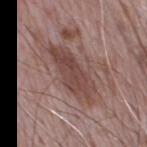No biopsy was performed on this lesion — it was imaged during a full skin examination and was not determined to be concerning. On the chest. About 8 mm across. An algorithmic analysis of the crop reported a mean CIELAB color near L≈46 a*≈19 b*≈21, a lesion–skin lightness drop of about 10, and a normalized border contrast of about 7.5. It also reported an automated nevus-likeness rating near 10 out of 100 and a lesion-detection confidence of about 80/100. This image is a 15 mm lesion crop taken from a total-body photograph. Captured under white-light illumination. A male subject, aged approximately 70.